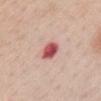<case>
<biopsy_status>not biopsied; imaged during a skin examination</biopsy_status>
<lighting>white-light</lighting>
<lesion_size>
  <long_diameter_mm_approx>2.5</long_diameter_mm_approx>
</lesion_size>
<site>chest</site>
<image>
  <source>total-body photography crop</source>
  <field_of_view_mm>15</field_of_view_mm>
</image>
<patient>
  <sex>female</sex>
  <age_approx>65</age_approx>
</patient>
</case>